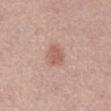Acquisition and patient details: A male subject aged 73–77. A region of skin cropped from a whole-body photographic capture, roughly 15 mm wide. Measured at roughly 3 mm in maximum diameter. On the abdomen. Automated image analysis of the tile measured a mean CIELAB color near L≈58 a*≈22 b*≈26 and a normalized lesion–skin contrast near 6.5. The analysis additionally found a within-lesion color-variation index near 2/10 and radial color variation of about 0.5.